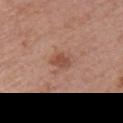Findings:
– workup: total-body-photography surveillance lesion; no biopsy
– site: the right upper arm
– lesion size: ≈2.5 mm
– tile lighting: white-light illumination
– subject: female, about 50 years old
– TBP lesion metrics: a mean CIELAB color near L≈49 a*≈23 b*≈30, a lesion–skin lightness drop of about 10, and a normalized lesion–skin contrast near 7.5; a color-variation rating of about 2/10 and radial color variation of about 0.5; an automated nevus-likeness rating near 65 out of 100
– image: total-body-photography crop, ~15 mm field of view